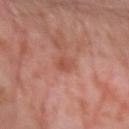Case summary:
* follow-up — catalogued during a skin exam; not biopsied
* diameter — ~2.5 mm (longest diameter)
* anatomic site — the left forearm
* tile lighting — cross-polarized illumination
* subject — female, aged 53 to 57
* image source — total-body-photography crop, ~15 mm field of view